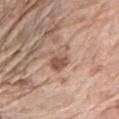notes — total-body-photography surveillance lesion; no biopsy | subject — female, aged approximately 75 | tile lighting — white-light | location — the right upper arm | size — ~4 mm (longest diameter) | image — ~15 mm crop, total-body skin-cancer survey.